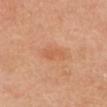Assessment:
Part of a total-body skin-imaging series; this lesion was reviewed on a skin check and was not flagged for biopsy.
Acquisition and patient details:
A close-up tile cropped from a whole-body skin photograph, about 15 mm across. The subject is a female in their 60s. Automated image analysis of the tile measured an area of roughly 4.5 mm², a shape eccentricity near 0.9, and a shape-asymmetry score of about 0.25 (0 = symmetric). The software also gave a nevus-likeness score of about 0/100 and a detector confidence of about 100 out of 100 that the crop contains a lesion. Imaged with cross-polarized lighting. The lesion's longest dimension is about 3.5 mm. Located on the head or neck.